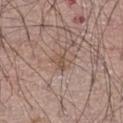biopsy status: imaged on a skin check; not biopsied | patient: male, in their mid- to late 60s | lighting: white-light | lesion diameter: about 2.5 mm | automated lesion analysis: a footprint of about 4 mm² and an eccentricity of roughly 0.7; a mean CIELAB color near L≈52 a*≈16 b*≈26, roughly 8 lightness units darker than nearby skin, and a lesion-to-skin contrast of about 6 (normalized; higher = more distinct); an automated nevus-likeness rating near 0 out of 100 and a lesion-detection confidence of about 85/100 | body site: the leg | image source: ~15 mm crop, total-body skin-cancer survey.